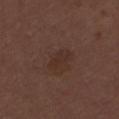site: the mid back | image: ~15 mm tile from a whole-body skin photo | subject: male, in their 30s | illumination: white-light | diameter: ≈3.5 mm | TBP lesion metrics: a mean CIELAB color near L≈29 a*≈17 b*≈22, a lesion–skin lightness drop of about 5, and a lesion-to-skin contrast of about 5.5 (normalized; higher = more distinct); a classifier nevus-likeness of about 10/100.